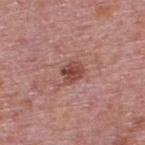  biopsy_status: not biopsied; imaged during a skin examination
  lesion_size:
    long_diameter_mm_approx: 2.5
  lighting: white-light
  patient:
    sex: male
    age_approx: 75
  image:
    source: total-body photography crop
    field_of_view_mm: 15
  site: upper back
  automated_metrics:
    border_irregularity_0_10: 2.0
    peripheral_color_asymmetry: 1.5